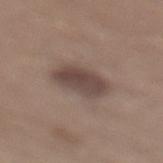Q: Is there a histopathology result?
A: no biopsy performed (imaged during a skin exam)
Q: What kind of image is this?
A: ~15 mm tile from a whole-body skin photo
Q: Automated lesion metrics?
A: a footprint of about 12 mm², a shape eccentricity near 0.8, and two-axis asymmetry of about 0.15; an average lesion color of about L≈44 a*≈15 b*≈20 (CIELAB) and roughly 11 lightness units darker than nearby skin
Q: How large is the lesion?
A: ~5 mm (longest diameter)
Q: Illumination type?
A: white-light
Q: Patient demographics?
A: male, about 30 years old
Q: Where on the body is the lesion?
A: the leg